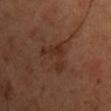Q: Is there a histopathology result?
A: total-body-photography surveillance lesion; no biopsy